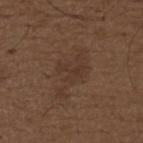Q: Was this lesion biopsied?
A: catalogued during a skin exam; not biopsied
Q: Lesion location?
A: the upper back
Q: What is the imaging modality?
A: total-body-photography crop, ~15 mm field of view
Q: Patient demographics?
A: male, roughly 50 years of age
Q: How large is the lesion?
A: about 3.5 mm
Q: Automated lesion metrics?
A: an outline eccentricity of about 0.85 (0 = round, 1 = elongated) and a shape-asymmetry score of about 0.65 (0 = symmetric); an average lesion color of about L≈33 a*≈16 b*≈24 (CIELAB) and roughly 5 lightness units darker than nearby skin
Q: Illumination type?
A: white-light